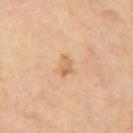lighting=cross-polarized
image source=total-body-photography crop, ~15 mm field of view
body site=the right upper arm
automated metrics=a border-irregularity rating of about 4.5/10, a color-variation rating of about 0/10, and radial color variation of about 0
lesion diameter=~3 mm (longest diameter)
patient=male, aged 63 to 67